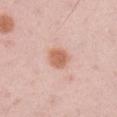{
  "biopsy_status": "not biopsied; imaged during a skin examination",
  "image": {
    "source": "total-body photography crop",
    "field_of_view_mm": 15
  },
  "site": "right upper arm",
  "lesion_size": {
    "long_diameter_mm_approx": 3.0
  },
  "lighting": "white-light",
  "patient": {
    "sex": "male",
    "age_approx": 35
  }
}